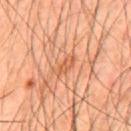A 15 mm close-up tile from a total-body photography series done for melanoma screening. Approximately 3 mm at its widest. This is a cross-polarized tile. An algorithmic analysis of the crop reported a lesion area of about 3.5 mm², an eccentricity of roughly 0.9, and a symmetry-axis asymmetry near 0.3. The analysis additionally found border irregularity of about 3 on a 0–10 scale, a color-variation rating of about 2/10, and radial color variation of about 0.5. The lesion is on the mid back. A male patient, approximately 45 years of age.